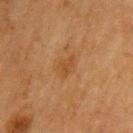A region of skin cropped from a whole-body photographic capture, roughly 15 mm wide. The tile uses cross-polarized illumination. The lesion is located on the left upper arm. A female patient, aged approximately 55. About 3 mm across.